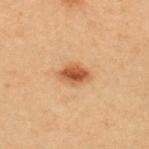Recorded during total-body skin imaging; not selected for excision or biopsy. A female patient, aged approximately 30. Longest diameter approximately 3.5 mm. From the upper back. A 15 mm close-up tile from a total-body photography series done for melanoma screening. The tile uses cross-polarized illumination.